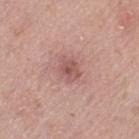biopsy status = no biopsy performed (imaged during a skin exam) | tile lighting = white-light illumination | automated metrics = a lesion color around L≈55 a*≈23 b*≈23 in CIELAB and a lesion–skin lightness drop of about 9; border irregularity of about 2.5 on a 0–10 scale and radial color variation of about 1 | patient = female, in their 40s | image = total-body-photography crop, ~15 mm field of view | location = the left thigh | size = ~3 mm (longest diameter).